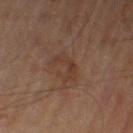{
  "biopsy_status": "not biopsied; imaged during a skin examination",
  "lesion_size": {
    "long_diameter_mm_approx": 3.0
  },
  "patient": {
    "sex": "male",
    "age_approx": 65
  },
  "site": "left leg",
  "image": {
    "source": "total-body photography crop",
    "field_of_view_mm": 15
  },
  "lighting": "cross-polarized",
  "automated_metrics": {
    "area_mm2_approx": 4.5,
    "eccentricity": 0.8,
    "shape_asymmetry": 0.65,
    "border_irregularity_0_10": 8.0,
    "color_variation_0_10": 0.0,
    "peripheral_color_asymmetry": 0.0
  }
}